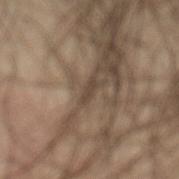Impression:
Part of a total-body skin-imaging series; this lesion was reviewed on a skin check and was not flagged for biopsy.
Background:
Captured under cross-polarized illumination. The lesion is located on the abdomen. This image is a 15 mm lesion crop taken from a total-body photograph. The lesion's longest dimension is about 2 mm. An algorithmic analysis of the crop reported a footprint of about 1.5 mm², a shape eccentricity near 0.95, and a symmetry-axis asymmetry near 0.45. It also reported a lesion–skin lightness drop of about 8. The software also gave a border-irregularity index near 4.5/10 and internal color variation of about 0 on a 0–10 scale. A male patient, aged around 40.